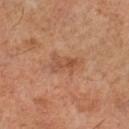Q: Was this lesion biopsied?
A: no biopsy performed (imaged during a skin exam)
Q: Illumination type?
A: cross-polarized
Q: How large is the lesion?
A: ~3.5 mm (longest diameter)
Q: Lesion location?
A: the left lower leg
Q: Who is the patient?
A: male, about 60 years old
Q: What kind of image is this?
A: total-body-photography crop, ~15 mm field of view